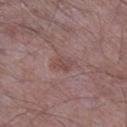Assessment:
Recorded during total-body skin imaging; not selected for excision or biopsy.
Clinical summary:
The lesion is located on the right lower leg. Automated tile analysis of the lesion measured a lesion area of about 5 mm². The software also gave a nevus-likeness score of about 0/100 and lesion-presence confidence of about 100/100. A male subject aged 48 to 52. Approximately 3 mm at its widest. A region of skin cropped from a whole-body photographic capture, roughly 15 mm wide.